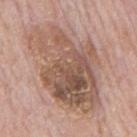Impression: Captured during whole-body skin photography for melanoma surveillance; the lesion was not biopsied. Background: Measured at roughly 10.5 mm in maximum diameter. The lesion is located on the mid back. A 15 mm close-up tile from a total-body photography series done for melanoma screening. A male patient approximately 75 years of age.